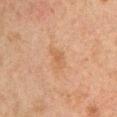Clinical impression: The lesion was tiled from a total-body skin photograph and was not biopsied. Acquisition and patient details: Measured at roughly 3 mm in maximum diameter. The lesion is located on the arm. A close-up tile cropped from a whole-body skin photograph, about 15 mm across. The subject is a male aged approximately 50. Captured under cross-polarized illumination.